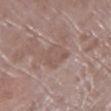Image and clinical context:
The lesion is located on the leg. A female subject, about 70 years old. Captured under white-light illumination. The lesion's longest dimension is about 3.5 mm. A close-up tile cropped from a whole-body skin photograph, about 15 mm across.